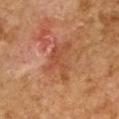Case summary:
– notes · no biopsy performed (imaged during a skin exam)
– imaging modality · total-body-photography crop, ~15 mm field of view
– lighting · cross-polarized illumination
– location · the right upper arm
– lesion diameter · ~5 mm (longest diameter)
– automated lesion analysis · a lesion–skin lightness drop of about 7 and a normalized border contrast of about 5.5; border irregularity of about 8.5 on a 0–10 scale, a color-variation rating of about 1.5/10, and radial color variation of about 0.5
– patient · female, aged 58–62